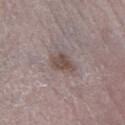Case summary:
- biopsy status: imaged on a skin check; not biopsied
- image source: ~15 mm tile from a whole-body skin photo
- subject: male, approximately 70 years of age
- diameter: about 3.5 mm
- tile lighting: white-light
- anatomic site: the right lower leg